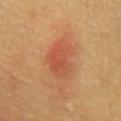workup: no biopsy performed (imaged during a skin exam)
lesion diameter: about 5 mm
image-analysis metrics: an area of roughly 11 mm² and an outline eccentricity of about 0.8 (0 = round, 1 = elongated); a classifier nevus-likeness of about 95/100 and lesion-presence confidence of about 100/100
site: the chest
patient: male, approximately 60 years of age
image: ~15 mm tile from a whole-body skin photo